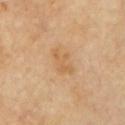{
  "biopsy_status": "not biopsied; imaged during a skin examination",
  "patient": {
    "sex": "female",
    "age_approx": 65
  },
  "image": {
    "source": "total-body photography crop",
    "field_of_view_mm": 15
  },
  "site": "left upper arm"
}